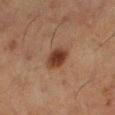{
  "biopsy_status": "not biopsied; imaged during a skin examination",
  "site": "left lower leg",
  "patient": {
    "sex": "female",
    "age_approx": 50
  },
  "image": {
    "source": "total-body photography crop",
    "field_of_view_mm": 15
  }
}